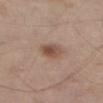biopsy status=no biopsy performed (imaged during a skin exam)
automated lesion analysis=an average lesion color of about L≈51 a*≈18 b*≈26 (CIELAB), a lesion–skin lightness drop of about 10, and a normalized border contrast of about 7.5; a border-irregularity rating of about 1.5/10, internal color variation of about 5 on a 0–10 scale, and radial color variation of about 1.5; a classifier nevus-likeness of about 85/100 and lesion-presence confidence of about 100/100
patient=male, roughly 75 years of age
image source=~15 mm crop, total-body skin-cancer survey
lighting=white-light illumination
diameter=about 3.5 mm
site=the right thigh